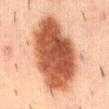Captured during whole-body skin photography for melanoma surveillance; the lesion was not biopsied.
Approximately 10.5 mm at its widest.
The tile uses cross-polarized illumination.
On the mid back.
A close-up tile cropped from a whole-body skin photograph, about 15 mm across.
Automated image analysis of the tile measured a footprint of about 50 mm² and an outline eccentricity of about 0.8 (0 = round, 1 = elongated).
The patient is a male about 55 years old.